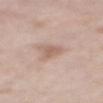{"biopsy_status": "not biopsied; imaged during a skin examination", "patient": {"sex": "male", "age_approx": 60}, "image": {"source": "total-body photography crop", "field_of_view_mm": 15}, "site": "back"}